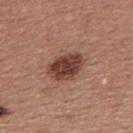workup: total-body-photography surveillance lesion; no biopsy
subject: male, in their 40s
location: the back
image source: 15 mm crop, total-body photography